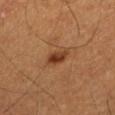Q: Was a biopsy performed?
A: catalogued during a skin exam; not biopsied
Q: What is the imaging modality?
A: ~15 mm tile from a whole-body skin photo
Q: Where on the body is the lesion?
A: the left thigh
Q: Patient demographics?
A: male, roughly 60 years of age